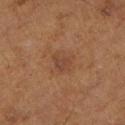biopsy status: no biopsy performed (imaged during a skin exam) | body site: the left lower leg | patient: male, in their mid- to late 70s | lighting: cross-polarized illumination | automated lesion analysis: a shape eccentricity near 0.55 and a shape-asymmetry score of about 0.4 (0 = symmetric); a border-irregularity rating of about 4/10, a within-lesion color-variation index near 3/10, and radial color variation of about 1; a nevus-likeness score of about 0/100 and lesion-presence confidence of about 100/100 | image: 15 mm crop, total-body photography.